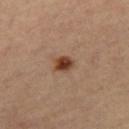Q: Was this lesion biopsied?
A: no biopsy performed (imaged during a skin exam)
Q: What is the imaging modality?
A: ~15 mm crop, total-body skin-cancer survey
Q: What are the patient's age and sex?
A: female, aged around 65
Q: Lesion location?
A: the leg
Q: Lesion size?
A: ~2 mm (longest diameter)